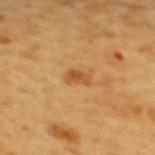No biopsy was performed on this lesion — it was imaged during a full skin examination and was not determined to be concerning. This is a cross-polarized tile. The patient is a female roughly 55 years of age. The lesion is located on the upper back. A 15 mm close-up tile from a total-body photography series done for melanoma screening.